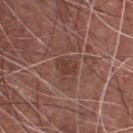The lesion was photographed on a routine skin check and not biopsied; there is no pathology result. Located on the chest. Captured under white-light illumination. The lesion-visualizer software estimated an area of roughly 4 mm² and a shape-asymmetry score of about 0.3 (0 = symmetric). And it measured a mean CIELAB color near L≈39 a*≈21 b*≈25, roughly 7 lightness units darker than nearby skin, and a normalized lesion–skin contrast near 6.5. A male patient in their mid-60s. Cropped from a whole-body photographic skin survey; the tile spans about 15 mm. About 2.5 mm across.